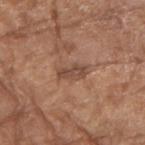No biopsy was performed on this lesion — it was imaged during a full skin examination and was not determined to be concerning.
The total-body-photography lesion software estimated a footprint of about 5 mm² and a shape-asymmetry score of about 0.25 (0 = symmetric). And it measured a border-irregularity rating of about 3/10. It also reported a nevus-likeness score of about 0/100 and a lesion-detection confidence of about 100/100.
A close-up tile cropped from a whole-body skin photograph, about 15 mm across.
This is a white-light tile.
The lesion is located on the left forearm.
The subject is a female roughly 75 years of age.
The recorded lesion diameter is about 3.5 mm.